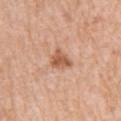Q: Patient demographics?
A: female, about 55 years old
Q: How was this image acquired?
A: total-body-photography crop, ~15 mm field of view
Q: What lighting was used for the tile?
A: white-light
Q: What did automated image analysis measure?
A: an eccentricity of roughly 0.5 and a shape-asymmetry score of about 0.35 (0 = symmetric); a nevus-likeness score of about 75/100 and a detector confidence of about 100 out of 100 that the crop contains a lesion
Q: Lesion location?
A: the left upper arm
Q: Lesion size?
A: ≈3 mm